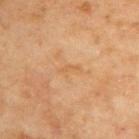follow-up = no biopsy performed (imaged during a skin exam) | tile lighting = cross-polarized illumination | TBP lesion metrics = a lesion color around L≈61 a*≈21 b*≈41 in CIELAB, roughly 5 lightness units darker than nearby skin, and a normalized lesion–skin contrast near 4.5; border irregularity of about 5 on a 0–10 scale, a color-variation rating of about 0/10, and peripheral color asymmetry of about 0; an automated nevus-likeness rating near 0 out of 100 and a lesion-detection confidence of about 100/100 | site = the upper back | image = total-body-photography crop, ~15 mm field of view | diameter = ≈2.5 mm | patient = male, aged around 70.